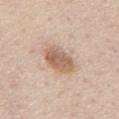workup: catalogued during a skin exam; not biopsied | image: total-body-photography crop, ~15 mm field of view | automated metrics: border irregularity of about 2 on a 0–10 scale and a color-variation rating of about 3.5/10 | site: the back | subject: male, approximately 60 years of age.